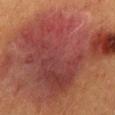Q: Is there a histopathology result?
A: total-body-photography surveillance lesion; no biopsy
Q: Who is the patient?
A: male, roughly 40 years of age
Q: Lesion location?
A: the back
Q: How was this image acquired?
A: total-body-photography crop, ~15 mm field of view